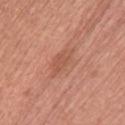No biopsy was performed on this lesion — it was imaged during a full skin examination and was not determined to be concerning.
From the upper back.
A region of skin cropped from a whole-body photographic capture, roughly 15 mm wide.
Measured at roughly 5 mm in maximum diameter.
The patient is a female roughly 60 years of age.
Imaged with white-light lighting.
The lesion-visualizer software estimated a mean CIELAB color near L≈55 a*≈25 b*≈31, roughly 8 lightness units darker than nearby skin, and a normalized border contrast of about 5.5. The analysis additionally found a within-lesion color-variation index near 2/10 and a peripheral color-asymmetry measure near 0.5.